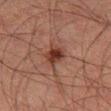Assessment: This lesion was catalogued during total-body skin photography and was not selected for biopsy. Image and clinical context: From the right thigh. Cropped from a total-body skin-imaging series; the visible field is about 15 mm. The subject is a male approximately 50 years of age.